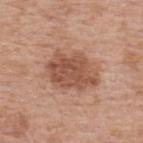workup = catalogued during a skin exam; not biopsied
lighting = white-light illumination
size = ≈6 mm
image source = 15 mm crop, total-body photography
subject = male, approximately 55 years of age
anatomic site = the upper back